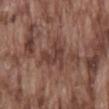Q: Was this lesion biopsied?
A: total-body-photography surveillance lesion; no biopsy
Q: Where on the body is the lesion?
A: the mid back
Q: What is the imaging modality?
A: ~15 mm tile from a whole-body skin photo
Q: How was the tile lit?
A: white-light
Q: What are the patient's age and sex?
A: male, in their mid- to late 70s
Q: How large is the lesion?
A: about 4.5 mm